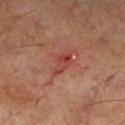No biopsy was performed on this lesion — it was imaged during a full skin examination and was not determined to be concerning. A male patient aged around 60. Longest diameter approximately 4 mm. On the left lower leg. This is a cross-polarized tile. A 15 mm close-up tile from a total-body photography series done for melanoma screening. The total-body-photography lesion software estimated a mean CIELAB color near L≈41 a*≈29 b*≈26, roughly 7 lightness units darker than nearby skin, and a lesion-to-skin contrast of about 6 (normalized; higher = more distinct).